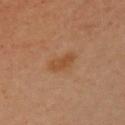{"automated_metrics": {"area_mm2_approx": 5.5, "eccentricity": 0.85, "shape_asymmetry": 0.25, "nevus_likeness_0_100": 25}, "lesion_size": {"long_diameter_mm_approx": 3.5}, "patient": {"sex": "male", "age_approx": 40}, "lighting": "cross-polarized", "image": {"source": "total-body photography crop", "field_of_view_mm": 15}, "site": "chest"}